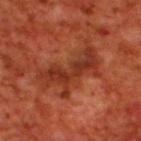biopsy_status: not biopsied; imaged during a skin examination